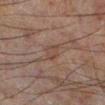Clinical impression: No biopsy was performed on this lesion — it was imaged during a full skin examination and was not determined to be concerning. Acquisition and patient details: A 15 mm close-up tile from a total-body photography series done for melanoma screening. Located on the left lower leg. The recorded lesion diameter is about 2.5 mm. This is a cross-polarized tile. The subject is a male approximately 60 years of age.